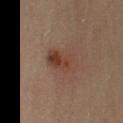workup — catalogued during a skin exam; not biopsied | image — 15 mm crop, total-body photography | subject — male, aged approximately 55 | location — the left upper arm.